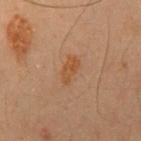No biopsy was performed on this lesion — it was imaged during a full skin examination and was not determined to be concerning.
Cropped from a whole-body photographic skin survey; the tile spans about 15 mm.
A male subject, aged approximately 50.
The lesion is on the chest.